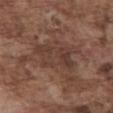Part of a total-body skin-imaging series; this lesion was reviewed on a skin check and was not flagged for biopsy. A 15 mm close-up extracted from a 3D total-body photography capture. Imaged with white-light lighting. The lesion is located on the abdomen. The lesion's longest dimension is about 6 mm. A male patient, in their mid-70s.